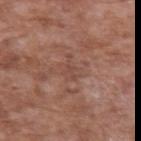Findings:
* notes: imaged on a skin check; not biopsied
* tile lighting: white-light illumination
* patient: male, aged around 60
* location: the left upper arm
* image: total-body-photography crop, ~15 mm field of view
* diameter: ≈3 mm
* automated lesion analysis: a lesion area of about 4 mm² and two-axis asymmetry of about 0.5; about 6 CIELAB-L* units darker than the surrounding skin and a lesion-to-skin contrast of about 4.5 (normalized; higher = more distinct); a nevus-likeness score of about 0/100 and a lesion-detection confidence of about 55/100